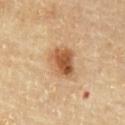Case summary:
• biopsy status — total-body-photography surveillance lesion; no biopsy
• lesion diameter — about 4 mm
• site — the chest
• patient — male, in their mid- to late 80s or older
• image source — total-body-photography crop, ~15 mm field of view